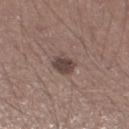Findings:
• follow-up · total-body-photography surveillance lesion; no biopsy
• tile lighting · white-light
• body site · the left lower leg
• acquisition · 15 mm crop, total-body photography
• subject · male, in their mid-40s